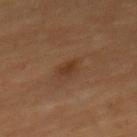Assessment:
Recorded during total-body skin imaging; not selected for excision or biopsy.
Background:
A roughly 15 mm field-of-view crop from a total-body skin photograph. The tile uses cross-polarized illumination. A male subject approximately 60 years of age. The lesion is located on the mid back.